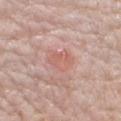{"biopsy_status": "not biopsied; imaged during a skin examination", "image": {"source": "total-body photography crop", "field_of_view_mm": 15}, "patient": {"sex": "male", "age_approx": 75}, "lesion_size": {"long_diameter_mm_approx": 3.5}, "site": "right upper arm"}